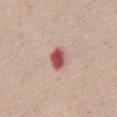notes = imaged on a skin check; not biopsied
tile lighting = white-light
subject = female, aged approximately 50
diameter = about 3 mm
anatomic site = the front of the torso
image source = ~15 mm tile from a whole-body skin photo